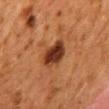No biopsy was performed on this lesion — it was imaged during a full skin examination and was not determined to be concerning.
Captured under cross-polarized illumination.
A female subject, aged 48 to 52.
The recorded lesion diameter is about 4 mm.
The total-body-photography lesion software estimated an area of roughly 9.5 mm² and two-axis asymmetry of about 0.25. The analysis additionally found a mean CIELAB color near L≈30 a*≈22 b*≈29, about 13 CIELAB-L* units darker than the surrounding skin, and a normalized border contrast of about 12. And it measured a classifier nevus-likeness of about 90/100 and lesion-presence confidence of about 100/100.
The lesion is on the mid back.
This image is a 15 mm lesion crop taken from a total-body photograph.